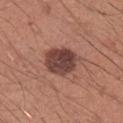The lesion was tiled from a total-body skin photograph and was not biopsied.
A region of skin cropped from a whole-body photographic capture, roughly 15 mm wide.
Captured under white-light illumination.
A male patient, aged 33 to 37.
The lesion is on the right upper arm.
Approximately 4 mm at its widest.